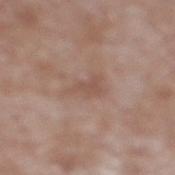{"site": "right lower leg", "patient": {"sex": "male", "age_approx": 60}, "image": {"source": "total-body photography crop", "field_of_view_mm": 15}, "lesion_size": {"long_diameter_mm_approx": 4.5}, "lighting": "white-light"}